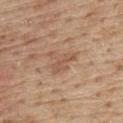Part of a total-body skin-imaging series; this lesion was reviewed on a skin check and was not flagged for biopsy. A male patient in their 70s. The tile uses white-light illumination. This image is a 15 mm lesion crop taken from a total-body photograph. The lesion is located on the chest. Automated tile analysis of the lesion measured a lesion area of about 6.5 mm², an outline eccentricity of about 0.6 (0 = round, 1 = elongated), and two-axis asymmetry of about 0.5. The analysis additionally found a mean CIELAB color near L≈55 a*≈20 b*≈30, roughly 8 lightness units darker than nearby skin, and a normalized border contrast of about 5.5.